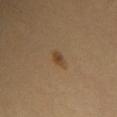subject — female, aged 28–32
imaging modality — ~15 mm crop, total-body skin-cancer survey
site — the chest
diameter — ~2.5 mm (longest diameter)